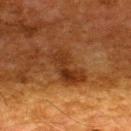Notes:
- follow-up: total-body-photography surveillance lesion; no biopsy
- imaging modality: ~15 mm tile from a whole-body skin photo
- body site: the back
- size: ≈5.5 mm
- subject: male, about 65 years old